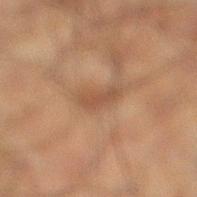Part of a total-body skin-imaging series; this lesion was reviewed on a skin check and was not flagged for biopsy. An algorithmic analysis of the crop reported a footprint of about 4 mm², an outline eccentricity of about 0.95 (0 = round, 1 = elongated), and a shape-asymmetry score of about 0.3 (0 = symmetric). A 15 mm close-up extracted from a 3D total-body photography capture. A male subject, roughly 50 years of age. Located on the right lower leg. Imaged with cross-polarized lighting. Measured at roughly 3.5 mm in maximum diameter.